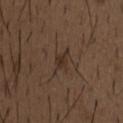No biopsy was performed on this lesion — it was imaged during a full skin examination and was not determined to be concerning.
The lesion's longest dimension is about 3 mm.
Located on the chest.
Automated tile analysis of the lesion measured a footprint of about 4.5 mm² and a shape-asymmetry score of about 0.45 (0 = symmetric). And it measured an average lesion color of about L≈31 a*≈14 b*≈23 (CIELAB) and a lesion-to-skin contrast of about 6.5 (normalized; higher = more distinct).
The tile uses white-light illumination.
The patient is a male aged around 50.
A 15 mm crop from a total-body photograph taken for skin-cancer surveillance.